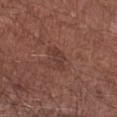Clinical impression: No biopsy was performed on this lesion — it was imaged during a full skin examination and was not determined to be concerning. Background: A male patient aged 63 to 67. The lesion is located on the right lower leg. A 15 mm crop from a total-body photograph taken for skin-cancer surveillance.